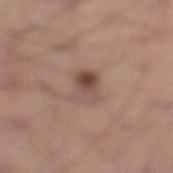{
  "biopsy_status": "not biopsied; imaged during a skin examination",
  "image": {
    "source": "total-body photography crop",
    "field_of_view_mm": 15
  },
  "patient": {
    "sex": "male",
    "age_approx": 30
  },
  "lesion_size": {
    "long_diameter_mm_approx": 4.0
  },
  "site": "left lower leg"
}